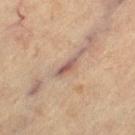Acquisition and patient details:
The recorded lesion diameter is about 3 mm. Imaged with cross-polarized lighting. Located on the leg. A female patient, in their mid-60s. Cropped from a whole-body photographic skin survey; the tile spans about 15 mm.